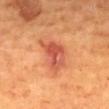Impression: No biopsy was performed on this lesion — it was imaged during a full skin examination and was not determined to be concerning. Background: A male subject, approximately 55 years of age. A 15 mm close-up tile from a total-body photography series done for melanoma screening. This is a cross-polarized tile. The lesion's longest dimension is about 4.5 mm. Automated image analysis of the tile measured an average lesion color of about L≈51 a*≈32 b*≈34 (CIELAB), a lesion–skin lightness drop of about 10, and a normalized lesion–skin contrast near 8. The lesion is on the mid back.